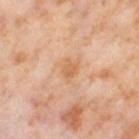workup: no biopsy performed (imaged during a skin exam) | body site: the right thigh | automated metrics: a mean CIELAB color near L≈64 a*≈23 b*≈38 and about 8 CIELAB-L* units darker than the surrounding skin | subject: female, aged 53 to 57 | image: total-body-photography crop, ~15 mm field of view.